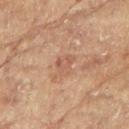biopsy_status: not biopsied; imaged during a skin examination
site: left arm
image:
  source: total-body photography crop
  field_of_view_mm: 15
patient:
  sex: female
  age_approx: 80